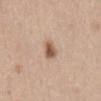| feature | finding |
|---|---|
| notes | total-body-photography surveillance lesion; no biopsy |
| patient | female, in their 40s |
| automated lesion analysis | a footprint of about 4 mm² and two-axis asymmetry of about 0.25; a mean CIELAB color near L≈56 a*≈19 b*≈30 and a lesion-to-skin contrast of about 9 (normalized; higher = more distinct); peripheral color asymmetry of about 1 |
| diameter | ~2.5 mm (longest diameter) |
| image | 15 mm crop, total-body photography |
| illumination | white-light |
| anatomic site | the abdomen |